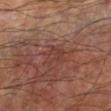patient=male, in their 60s | illumination=cross-polarized | lesion diameter=≈3 mm | site=the left lower leg | acquisition=total-body-photography crop, ~15 mm field of view.